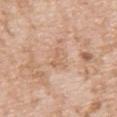Clinical impression:
Recorded during total-body skin imaging; not selected for excision or biopsy.
Image and clinical context:
A male patient aged around 50. The lesion is on the chest. A lesion tile, about 15 mm wide, cut from a 3D total-body photograph.